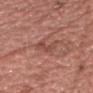Recorded during total-body skin imaging; not selected for excision or biopsy. The lesion is located on the front of the torso. A male subject roughly 75 years of age. The lesion's longest dimension is about 3 mm. The tile uses white-light illumination. A roughly 15 mm field-of-view crop from a total-body skin photograph.